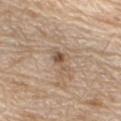Clinical impression: Recorded during total-body skin imaging; not selected for excision or biopsy. Clinical summary: The lesion is on the right upper arm. The patient is a male roughly 70 years of age. A 15 mm close-up tile from a total-body photography series done for melanoma screening.